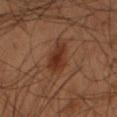This lesion was catalogued during total-body skin photography and was not selected for biopsy.
A roughly 15 mm field-of-view crop from a total-body skin photograph.
The lesion is located on the right forearm.
This is a cross-polarized tile.
The patient is a male approximately 55 years of age.
Approximately 3.5 mm at its widest.